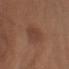<lesion>
  <biopsy_status>not biopsied; imaged during a skin examination</biopsy_status>
  <image>
    <source>total-body photography crop</source>
    <field_of_view_mm>15</field_of_view_mm>
  </image>
  <site>left upper arm</site>
  <patient>
    <sex>male</sex>
    <age_approx>75</age_approx>
  </patient>
</lesion>